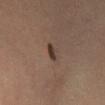{
  "biopsy_status": "not biopsied; imaged during a skin examination",
  "patient": {
    "sex": "female",
    "age_approx": 50
  },
  "image": {
    "source": "total-body photography crop",
    "field_of_view_mm": 15
  },
  "site": "leg",
  "lighting": "cross-polarized"
}